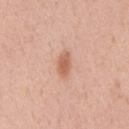biopsy status = total-body-photography surveillance lesion; no biopsy | location = the chest | patient = male, aged 53–57 | image = 15 mm crop, total-body photography | size = about 3 mm | image-analysis metrics = an average lesion color of about L≈61 a*≈24 b*≈32 (CIELAB), about 11 CIELAB-L* units darker than the surrounding skin, and a normalized lesion–skin contrast near 7.5; a border-irregularity index near 2/10, a color-variation rating of about 2/10, and a peripheral color-asymmetry measure near 1 | lighting = white-light illumination.